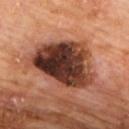Captured during whole-body skin photography for melanoma surveillance; the lesion was not biopsied.
A 15 mm crop from a total-body photograph taken for skin-cancer surveillance.
The lesion is located on the upper back.
Longest diameter approximately 7.5 mm.
A male patient, approximately 60 years of age.